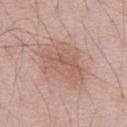notes = no biopsy performed (imaged during a skin exam); lesion diameter = ≈7 mm; patient = male, roughly 55 years of age; image = ~15 mm crop, total-body skin-cancer survey; site = the abdomen; illumination = white-light.